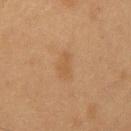Captured during whole-body skin photography for melanoma surveillance; the lesion was not biopsied. A roughly 15 mm field-of-view crop from a total-body skin photograph. From the chest. A male subject, roughly 55 years of age.